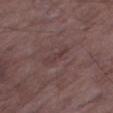biopsy status = no biopsy performed (imaged during a skin exam)
body site = the right thigh
patient = male, roughly 65 years of age
diameter = ≈3.5 mm
tile lighting = white-light illumination
acquisition = 15 mm crop, total-body photography